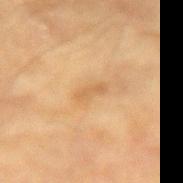<lesion>
<biopsy_status>not biopsied; imaged during a skin examination</biopsy_status>
<site>right upper arm</site>
<lighting>cross-polarized</lighting>
<lesion_size>
  <long_diameter_mm_approx>3.0</long_diameter_mm_approx>
</lesion_size>
<patient>
  <sex>male</sex>
  <age_approx>85+</age_approx>
</patient>
<image>
  <source>total-body photography crop</source>
  <field_of_view_mm>15</field_of_view_mm>
</image>
</lesion>